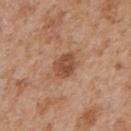No biopsy was performed on this lesion — it was imaged during a full skin examination and was not determined to be concerning. A lesion tile, about 15 mm wide, cut from a 3D total-body photograph. Located on the chest. The subject is a male in their mid-60s.